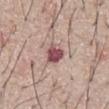automated metrics: a lesion area of about 5 mm², a shape eccentricity near 0.65, and a shape-asymmetry score of about 0.25 (0 = symmetric); a mean CIELAB color near L≈50 a*≈26 b*≈18, about 17 CIELAB-L* units darker than the surrounding skin, and a normalized lesion–skin contrast near 12; a border-irregularity index near 2.5/10 and internal color variation of about 5 on a 0–10 scale; an automated nevus-likeness rating near 5 out of 100 and lesion-presence confidence of about 100/100
anatomic site: the abdomen
diameter: ~3 mm (longest diameter)
lighting: white-light
image source: total-body-photography crop, ~15 mm field of view
subject: male, in their mid-60s